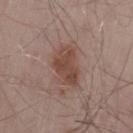follow-up: catalogued during a skin exam; not biopsied
location: the leg
acquisition: ~15 mm tile from a whole-body skin photo
patient: male, about 55 years old